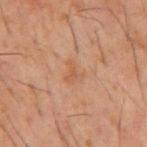  biopsy_status: not biopsied; imaged during a skin examination
  automated_metrics:
    area_mm2_approx: 4.5
    eccentricity: 0.65
    shape_asymmetry: 0.4
    cielab_L: 53
    cielab_a: 21
    cielab_b: 33
    vs_skin_darker_L: 5.0
    vs_skin_contrast_norm: 4.5
  lighting: cross-polarized
  site: mid back
  image:
    source: total-body photography crop
    field_of_view_mm: 15
  patient:
    sex: male
    age_approx: 60
  lesion_size:
    long_diameter_mm_approx: 2.5